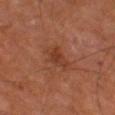The lesion was tiled from a total-body skin photograph and was not biopsied.
Captured under cross-polarized illumination.
On the left thigh.
A lesion tile, about 15 mm wide, cut from a 3D total-body photograph.
A male patient, in their mid- to late 60s.